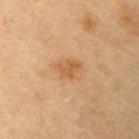Clinical summary: A roughly 15 mm field-of-view crop from a total-body skin photograph. This is a cross-polarized tile. Approximately 2.5 mm at its widest. The patient is a male aged around 85 or older. Located on the left upper arm. Automated tile analysis of the lesion measured an area of roughly 3.5 mm², a shape eccentricity near 0.6, and a shape-asymmetry score of about 0.35 (0 = symmetric). And it measured a lesion color around L≈56 a*≈21 b*≈39 in CIELAB, a lesion–skin lightness drop of about 8, and a lesion-to-skin contrast of about 6.5 (normalized; higher = more distinct). It also reported border irregularity of about 3.5 on a 0–10 scale, internal color variation of about 1.5 on a 0–10 scale, and peripheral color asymmetry of about 0.5. The software also gave a classifier nevus-likeness of about 20/100 and lesion-presence confidence of about 100/100.